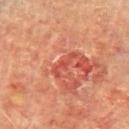Findings:
- site — the chest
- diameter — ≈4 mm
- patient — male, approximately 75 years of age
- lighting — cross-polarized illumination
- TBP lesion metrics — a lesion area of about 3.5 mm², an eccentricity of roughly 0.95, and a shape-asymmetry score of about 0.5 (0 = symmetric); a lesion–skin lightness drop of about 8 and a lesion-to-skin contrast of about 6 (normalized; higher = more distinct); a border-irregularity index near 7.5/10 and internal color variation of about 0 on a 0–10 scale; a detector confidence of about 70 out of 100 that the crop contains a lesion
- image — 15 mm crop, total-body photography